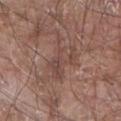Notes:
– notes · no biopsy performed (imaged during a skin exam)
– size · ≈7 mm
– subject · male, approximately 80 years of age
– anatomic site · the arm
– image · 15 mm crop, total-body photography
– lighting · white-light